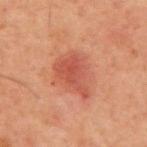{"biopsy_status": "not biopsied; imaged during a skin examination", "lighting": "cross-polarized", "image": {"source": "total-body photography crop", "field_of_view_mm": 15}, "lesion_size": {"long_diameter_mm_approx": 5.0}, "automated_metrics": {"cielab_L": 47, "cielab_a": 29, "cielab_b": 30, "vs_skin_contrast_norm": 6.5, "nevus_likeness_0_100": 70, "lesion_detection_confidence_0_100": 100}, "patient": {"sex": "male", "age_approx": 60}, "site": "front of the torso"}